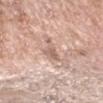Recorded during total-body skin imaging; not selected for excision or biopsy. Approximately 3.5 mm at its widest. The patient is a female about 75 years old. Imaged with white-light lighting. Automated image analysis of the tile measured a footprint of about 4 mm², an eccentricity of roughly 0.85, and two-axis asymmetry of about 0.55. And it measured about 10 CIELAB-L* units darker than the surrounding skin and a normalized lesion–skin contrast near 6. It also reported a classifier nevus-likeness of about 0/100. Cropped from a whole-body photographic skin survey; the tile spans about 15 mm. The lesion is located on the right forearm.